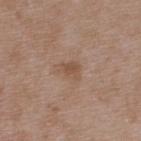Assessment:
The lesion was photographed on a routine skin check and not biopsied; there is no pathology result.
Context:
On the upper back. The patient is a male aged 48–52. A close-up tile cropped from a whole-body skin photograph, about 15 mm across. Measured at roughly 2.5 mm in maximum diameter.